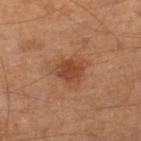The lesion was tiled from a total-body skin photograph and was not biopsied. Imaged with cross-polarized lighting. The recorded lesion diameter is about 3 mm. The lesion is on the right lower leg. Automated tile analysis of the lesion measured an area of roughly 6 mm², a shape eccentricity near 0.6, and a symmetry-axis asymmetry near 0.2. The analysis additionally found a lesion color around L≈42 a*≈24 b*≈32 in CIELAB and about 9 CIELAB-L* units darker than the surrounding skin. And it measured internal color variation of about 2.5 on a 0–10 scale and radial color variation of about 1. It also reported a nevus-likeness score of about 40/100. The patient is a male aged around 65. A 15 mm close-up tile from a total-body photography series done for melanoma screening.